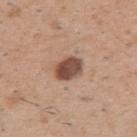notes: no biopsy performed (imaged during a skin exam) | lesion diameter: ≈3.5 mm | body site: the arm | illumination: white-light | TBP lesion metrics: a footprint of about 7.5 mm² and an outline eccentricity of about 0.6 (0 = round, 1 = elongated); an average lesion color of about L≈49 a*≈20 b*≈27 (CIELAB) and a normalized border contrast of about 11; a classifier nevus-likeness of about 60/100 and a lesion-detection confidence of about 100/100 | image: ~15 mm crop, total-body skin-cancer survey | patient: male, about 50 years old.